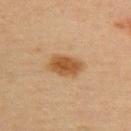{"biopsy_status": "not biopsied; imaged during a skin examination", "lighting": "cross-polarized", "lesion_size": {"long_diameter_mm_approx": 4.5}, "patient": {"sex": "female", "age_approx": 45}, "site": "upper back", "image": {"source": "total-body photography crop", "field_of_view_mm": 15}, "automated_metrics": {"area_mm2_approx": 10.0, "eccentricity": 0.8, "shape_asymmetry": 0.2, "nevus_likeness_0_100": 100, "lesion_detection_confidence_0_100": 100}}